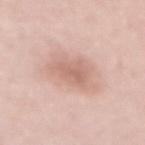| feature | finding |
|---|---|
| follow-up | catalogued during a skin exam; not biopsied |
| tile lighting | white-light illumination |
| site | the mid back |
| image-analysis metrics | an area of roughly 16 mm² and two-axis asymmetry of about 0.3; border irregularity of about 4 on a 0–10 scale and a peripheral color-asymmetry measure near 1; a lesion-detection confidence of about 100/100 |
| patient | female, aged 48–52 |
| imaging modality | ~15 mm crop, total-body skin-cancer survey |